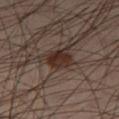The lesion was tiled from a total-body skin photograph and was not biopsied.
A male patient, aged approximately 40.
A region of skin cropped from a whole-body photographic capture, roughly 15 mm wide.
About 3 mm across.
On the right thigh.